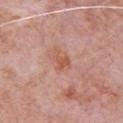image source = total-body-photography crop, ~15 mm field of view; patient = male, roughly 80 years of age; site = the chest.